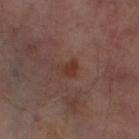Q: Is there a histopathology result?
A: no biopsy performed (imaged during a skin exam)
Q: Patient demographics?
A: male, in their mid- to late 60s
Q: Where on the body is the lesion?
A: the left thigh
Q: Lesion size?
A: ~2.5 mm (longest diameter)
Q: What did automated image analysis measure?
A: a lesion area of about 3.5 mm² and a shape-asymmetry score of about 0.4 (0 = symmetric); an average lesion color of about L≈32 a*≈21 b*≈24 (CIELAB) and a lesion-to-skin contrast of about 7.5 (normalized; higher = more distinct); a border-irregularity index near 4/10 and radial color variation of about 0.5; an automated nevus-likeness rating near 90 out of 100 and lesion-presence confidence of about 100/100
Q: What kind of image is this?
A: ~15 mm crop, total-body skin-cancer survey
Q: Illumination type?
A: cross-polarized illumination